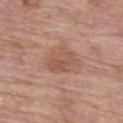<lesion>
<biopsy_status>not biopsied; imaged during a skin examination</biopsy_status>
<image>
  <source>total-body photography crop</source>
  <field_of_view_mm>15</field_of_view_mm>
</image>
<automated_metrics>
  <border_irregularity_0_10>2.0</border_irregularity_0_10>
  <color_variation_0_10>3.5</color_variation_0_10>
  <peripheral_color_asymmetry>1.0</peripheral_color_asymmetry>
</automated_metrics>
<patient>
  <sex>female</sex>
  <age_approx>85+</age_approx>
</patient>
<site>left thigh</site>
<lighting>white-light</lighting>
</lesion>